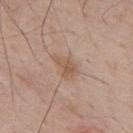The lesion was photographed on a routine skin check and not biopsied; there is no pathology result. Located on the back. The subject is a male aged approximately 70. Cropped from a total-body skin-imaging series; the visible field is about 15 mm. Imaged with white-light lighting.